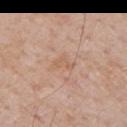Clinical impression:
Recorded during total-body skin imaging; not selected for excision or biopsy.
Context:
From the left upper arm. A roughly 15 mm field-of-view crop from a total-body skin photograph. The patient is a male roughly 70 years of age.